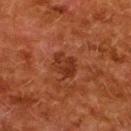Q: Was a biopsy performed?
A: no biopsy performed (imaged during a skin exam)
Q: What is the imaging modality?
A: ~15 mm crop, total-body skin-cancer survey
Q: What are the patient's age and sex?
A: female, roughly 50 years of age
Q: Where on the body is the lesion?
A: the back
Q: What did automated image analysis measure?
A: an area of roughly 6.5 mm², an outline eccentricity of about 0.6 (0 = round, 1 = elongated), and a shape-asymmetry score of about 0.2 (0 = symmetric); about 7 CIELAB-L* units darker than the surrounding skin and a normalized lesion–skin contrast near 7